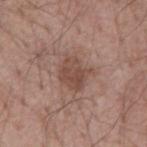Assessment: Captured during whole-body skin photography for melanoma surveillance; the lesion was not biopsied. Clinical summary: The lesion is on the mid back. Approximately 3.5 mm at its widest. A 15 mm close-up tile from a total-body photography series done for melanoma screening. The patient is a male aged 53 to 57.